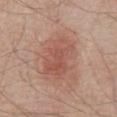Impression:
Imaged during a routine full-body skin examination; the lesion was not biopsied and no histopathology is available.
Clinical summary:
The tile uses white-light illumination. Longest diameter approximately 5.5 mm. A region of skin cropped from a whole-body photographic capture, roughly 15 mm wide. A male subject, roughly 80 years of age. The lesion is located on the abdomen.